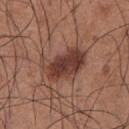{"biopsy_status": "not biopsied; imaged during a skin examination", "automated_metrics": {"area_mm2_approx": 15.0, "eccentricity": 0.8, "shape_asymmetry": 0.25, "border_irregularity_0_10": 3.5, "peripheral_color_asymmetry": 2.0, "nevus_likeness_0_100": 80}, "image": {"source": "total-body photography crop", "field_of_view_mm": 15}, "lighting": "white-light", "lesion_size": {"long_diameter_mm_approx": 5.5}, "site": "chest", "patient": {"sex": "male", "age_approx": 35}}